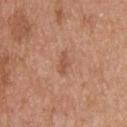Q: Was this lesion biopsied?
A: no biopsy performed (imaged during a skin exam)
Q: Lesion location?
A: the upper back
Q: How large is the lesion?
A: about 2.5 mm
Q: What kind of image is this?
A: ~15 mm tile from a whole-body skin photo
Q: Who is the patient?
A: male, approximately 60 years of age
Q: How was the tile lit?
A: white-light illumination